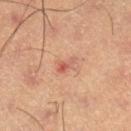<record>
  <biopsy_status>not biopsied; imaged during a skin examination</biopsy_status>
  <image>
    <source>total-body photography crop</source>
    <field_of_view_mm>15</field_of_view_mm>
  </image>
  <automated_metrics>
    <border_irregularity_0_10>3.5</border_irregularity_0_10>
    <nevus_likeness_0_100>0</nevus_likeness_0_100>
  </automated_metrics>
  <patient>
    <sex>male</sex>
    <age_approx>55</age_approx>
  </patient>
  <site>left lower leg</site>
  <lesion_size>
    <long_diameter_mm_approx>3.0</long_diameter_mm_approx>
  </lesion_size>
</record>